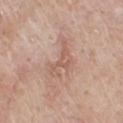– follow-up — imaged on a skin check; not biopsied
– subject — male, approximately 65 years of age
– body site — the chest
– size — ~5 mm (longest diameter)
– tile lighting — white-light illumination
– acquisition — total-body-photography crop, ~15 mm field of view